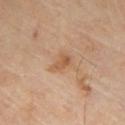{
  "biopsy_status": "not biopsied; imaged during a skin examination",
  "site": "front of the torso",
  "lighting": "cross-polarized",
  "image": {
    "source": "total-body photography crop",
    "field_of_view_mm": 15
  },
  "patient": {
    "sex": "male",
    "age_approx": 65
  }
}